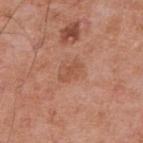A 15 mm close-up tile from a total-body photography series done for melanoma screening. A male patient, about 55 years old. The lesion is located on the back. The total-body-photography lesion software estimated a lesion–skin lightness drop of about 7 and a normalized lesion–skin contrast near 5.5. And it measured a nevus-likeness score of about 5/100 and a lesion-detection confidence of about 100/100.